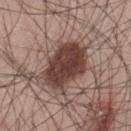follow-up = no biopsy performed (imaged during a skin exam) | patient = male, about 70 years old | illumination = white-light illumination | diameter = ≈6 mm | image source = 15 mm crop, total-body photography | body site = the lower back.